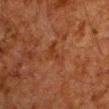Q: Is there a histopathology result?
A: no biopsy performed (imaged during a skin exam)
Q: Where on the body is the lesion?
A: the chest
Q: Patient demographics?
A: male, aged 58 to 62
Q: What is the lesion's diameter?
A: ≈3.5 mm
Q: Illumination type?
A: cross-polarized
Q: What kind of image is this?
A: total-body-photography crop, ~15 mm field of view